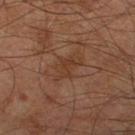– follow-up · no biopsy performed (imaged during a skin exam)
– acquisition · ~15 mm tile from a whole-body skin photo
– lesion diameter · about 4 mm
– patient · male, aged around 60
– lighting · cross-polarized illumination
– body site · the right leg
– automated metrics · a normalized border contrast of about 5.5; a border-irregularity rating of about 6/10 and radial color variation of about 0.5; a nevus-likeness score of about 0/100 and a lesion-detection confidence of about 100/100